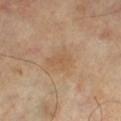Clinical impression: Captured during whole-body skin photography for melanoma surveillance; the lesion was not biopsied. Context: The subject is a female in their 60s. Captured under cross-polarized illumination. A close-up tile cropped from a whole-body skin photograph, about 15 mm across. On the right leg. The lesion-visualizer software estimated a footprint of about 6 mm², an outline eccentricity of about 0.65 (0 = round, 1 = elongated), and two-axis asymmetry of about 0.4. The software also gave a nevus-likeness score of about 0/100. Measured at roughly 3 mm in maximum diameter.